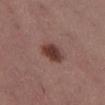Context: Automated image analysis of the tile measured a lesion area of about 7.5 mm² and a shape-asymmetry score of about 0.15 (0 = symmetric). And it measured an average lesion color of about L≈39 a*≈21 b*≈22 (CIELAB), roughly 12 lightness units darker than nearby skin, and a lesion-to-skin contrast of about 10 (normalized; higher = more distinct). It also reported a border-irregularity rating of about 1.5/10, a color-variation rating of about 3/10, and a peripheral color-asymmetry measure near 1. And it measured a classifier nevus-likeness of about 100/100. The lesion's longest dimension is about 3 mm. The lesion is located on the left thigh. A region of skin cropped from a whole-body photographic capture, roughly 15 mm wide. A female patient in their mid- to late 50s. Captured under white-light illumination.